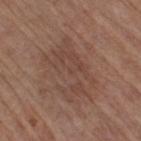The lesion was photographed on a routine skin check and not biopsied; there is no pathology result.
The recorded lesion diameter is about 7 mm.
A female subject, aged around 55.
A roughly 15 mm field-of-view crop from a total-body skin photograph.
On the right thigh.
This is a white-light tile.
An algorithmic analysis of the crop reported an average lesion color of about L≈44 a*≈19 b*≈24 (CIELAB) and roughly 6 lightness units darker than nearby skin. It also reported border irregularity of about 8.5 on a 0–10 scale, internal color variation of about 3 on a 0–10 scale, and radial color variation of about 1. And it measured a classifier nevus-likeness of about 0/100 and a detector confidence of about 100 out of 100 that the crop contains a lesion.